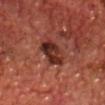No biopsy was performed on this lesion — it was imaged during a full skin examination and was not determined to be concerning. A region of skin cropped from a whole-body photographic capture, roughly 15 mm wide. On the chest. A male patient aged 68–72.